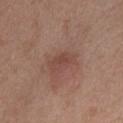Q: Automated lesion metrics?
A: a footprint of about 5 mm² and a shape eccentricity near 0.85; a normalized border contrast of about 5.5; an automated nevus-likeness rating near 80 out of 100 and a lesion-detection confidence of about 100/100
Q: What is the lesion's diameter?
A: ≈3 mm
Q: How was the tile lit?
A: white-light illumination
Q: Who is the patient?
A: female, aged approximately 40
Q: What is the anatomic site?
A: the upper back
Q: What is the imaging modality?
A: 15 mm crop, total-body photography